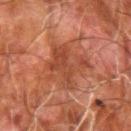This lesion was catalogued during total-body skin photography and was not selected for biopsy.